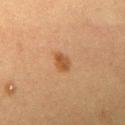Clinical impression: Part of a total-body skin-imaging series; this lesion was reviewed on a skin check and was not flagged for biopsy. Background: The subject is a female aged 38–42. An algorithmic analysis of the crop reported about 9 CIELAB-L* units darker than the surrounding skin and a lesion-to-skin contrast of about 8 (normalized; higher = more distinct). The software also gave an automated nevus-likeness rating near 95 out of 100 and lesion-presence confidence of about 100/100. This is a cross-polarized tile. A region of skin cropped from a whole-body photographic capture, roughly 15 mm wide. Located on the chest. Measured at roughly 2.5 mm in maximum diameter.